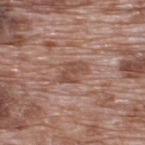Assessment: The lesion was photographed on a routine skin check and not biopsied; there is no pathology result. Background: This image is a 15 mm lesion crop taken from a total-body photograph. Measured at roughly 3.5 mm in maximum diameter. Automated image analysis of the tile measured a mean CIELAB color near L≈49 a*≈20 b*≈27. The analysis additionally found a classifier nevus-likeness of about 0/100 and lesion-presence confidence of about 90/100. Imaged with white-light lighting. A male patient, roughly 70 years of age. Located on the back.